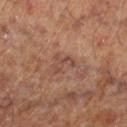Recorded during total-body skin imaging; not selected for excision or biopsy. A close-up tile cropped from a whole-body skin photograph, about 15 mm across. The lesion is on the right lower leg. Captured under cross-polarized illumination. A male patient, in their 60s.